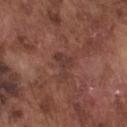Part of a total-body skin-imaging series; this lesion was reviewed on a skin check and was not flagged for biopsy. A male subject, in their mid-70s. The lesion's longest dimension is about 3.5 mm. A 15 mm crop from a total-body photograph taken for skin-cancer surveillance. On the chest. Imaged with white-light lighting.